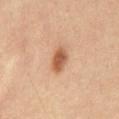biopsy status = total-body-photography surveillance lesion; no biopsy
subject = male, about 60 years old
location = the abdomen
tile lighting = cross-polarized illumination
image source = ~15 mm crop, total-body skin-cancer survey
automated lesion analysis = a symmetry-axis asymmetry near 0.25; a normalized lesion–skin contrast near 8.5; a border-irregularity rating of about 2.5/10, a within-lesion color-variation index near 3.5/10, and radial color variation of about 1